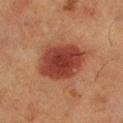Imaged during a routine full-body skin examination; the lesion was not biopsied and no histopathology is available. Measured at roughly 6 mm in maximum diameter. This is a cross-polarized tile. The lesion is on the left lower leg. This image is a 15 mm lesion crop taken from a total-body photograph. Automated tile analysis of the lesion measured a footprint of about 21 mm², an outline eccentricity of about 0.7 (0 = round, 1 = elongated), and two-axis asymmetry of about 0.15. The patient is a female aged 48 to 52.